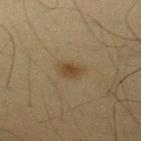This lesion was catalogued during total-body skin photography and was not selected for biopsy.
An algorithmic analysis of the crop reported an area of roughly 3.5 mm², an outline eccentricity of about 0.8 (0 = round, 1 = elongated), and two-axis asymmetry of about 0.25. The analysis additionally found a mean CIELAB color near L≈35 a*≈11 b*≈28, a lesion–skin lightness drop of about 7, and a lesion-to-skin contrast of about 8 (normalized; higher = more distinct).
A male patient, aged approximately 65.
The lesion is located on the arm.
Cropped from a total-body skin-imaging series; the visible field is about 15 mm.